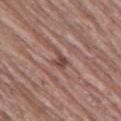Clinical impression:
Imaged during a routine full-body skin examination; the lesion was not biopsied and no histopathology is available.
Image and clinical context:
A male patient aged approximately 65. Measured at roughly 2.5 mm in maximum diameter. Imaged with white-light lighting. On the left thigh. A lesion tile, about 15 mm wide, cut from a 3D total-body photograph.